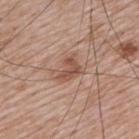| field | value |
|---|---|
| diameter | ~3 mm (longest diameter) |
| body site | the upper back |
| image | total-body-photography crop, ~15 mm field of view |
| subject | male, aged 63 to 67 |
| automated lesion analysis | an area of roughly 5 mm², a shape eccentricity near 0.7, and a symmetry-axis asymmetry near 0.45; a mean CIELAB color near L≈51 a*≈22 b*≈28 and a normalized border contrast of about 7; a border-irregularity index near 4/10, a within-lesion color-variation index near 3/10, and radial color variation of about 1; a classifier nevus-likeness of about 65/100 and lesion-presence confidence of about 100/100 |
| illumination | white-light illumination |